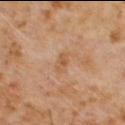Assessment: Recorded during total-body skin imaging; not selected for excision or biopsy. Acquisition and patient details: This is a cross-polarized tile. A lesion tile, about 15 mm wide, cut from a 3D total-body photograph. The subject is a male in their 60s. The lesion is located on the chest. The total-body-photography lesion software estimated a border-irregularity rating of about 2.5/10 and peripheral color asymmetry of about 0.5. Measured at roughly 2.5 mm in maximum diameter.